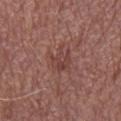Part of a total-body skin-imaging series; this lesion was reviewed on a skin check and was not flagged for biopsy. About 4 mm across. On the right lower leg. A male patient, aged approximately 75. Automated tile analysis of the lesion measured internal color variation of about 3 on a 0–10 scale and a peripheral color-asymmetry measure near 1. The software also gave an automated nevus-likeness rating near 0 out of 100 and lesion-presence confidence of about 100/100. A roughly 15 mm field-of-view crop from a total-body skin photograph.